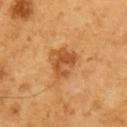biopsy status = catalogued during a skin exam; not biopsied
illumination = cross-polarized illumination
acquisition = ~15 mm crop, total-body skin-cancer survey
size = about 4 mm
patient = male, aged 58 to 62
TBP lesion metrics = a mean CIELAB color near L≈53 a*≈26 b*≈42 and a normalized lesion–skin contrast near 7; border irregularity of about 3.5 on a 0–10 scale, a color-variation rating of about 6.5/10, and a peripheral color-asymmetry measure near 2; a classifier nevus-likeness of about 80/100 and a lesion-detection confidence of about 100/100
body site = the right upper arm